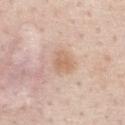Assessment: The lesion was tiled from a total-body skin photograph and was not biopsied. Image and clinical context: Captured under white-light illumination. The subject is a male about 60 years old. The lesion is located on the abdomen. About 2.5 mm across. A 15 mm crop from a total-body photograph taken for skin-cancer surveillance.